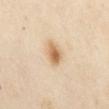Clinical impression:
The lesion was tiled from a total-body skin photograph and was not biopsied.
Context:
Longest diameter approximately 3 mm. The lesion is on the front of the torso. The total-body-photography lesion software estimated a lesion color around L≈67 a*≈17 b*≈37 in CIELAB and roughly 13 lightness units darker than nearby skin. The analysis additionally found a border-irregularity index near 2.5/10, a color-variation rating of about 4/10, and radial color variation of about 1.5. And it measured an automated nevus-likeness rating near 100 out of 100. A female patient, aged 53 to 57. This image is a 15 mm lesion crop taken from a total-body photograph. Captured under cross-polarized illumination.